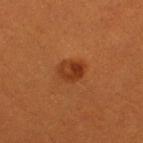biopsy_status: not biopsied; imaged during a skin examination
site: right thigh
lighting: cross-polarized
automated_metrics:
  cielab_L: 34
  cielab_a: 26
  cielab_b: 36
  vs_skin_darker_L: 9.0
  vs_skin_contrast_norm: 8.0
  nevus_likeness_0_100: 95
  lesion_detection_confidence_0_100: 100
image:
  source: total-body photography crop
  field_of_view_mm: 15
patient:
  sex: female
  age_approx: 30
lesion_size:
  long_diameter_mm_approx: 3.0